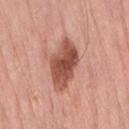Q: Was a biopsy performed?
A: no biopsy performed (imaged during a skin exam)
Q: Illumination type?
A: white-light
Q: Patient demographics?
A: female, aged 68 to 72
Q: Lesion size?
A: ~6 mm (longest diameter)
Q: What did automated image analysis measure?
A: a lesion-detection confidence of about 100/100
Q: What kind of image is this?
A: ~15 mm tile from a whole-body skin photo
Q: Where on the body is the lesion?
A: the lower back